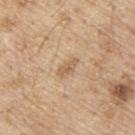workup: no biopsy performed (imaged during a skin exam) | automated metrics: an average lesion color of about L≈60 a*≈16 b*≈34 (CIELAB), a lesion–skin lightness drop of about 9, and a lesion-to-skin contrast of about 6 (normalized; higher = more distinct); a classifier nevus-likeness of about 0/100 | patient: male, aged approximately 70 | image source: ~15 mm tile from a whole-body skin photo | illumination: white-light | diameter: about 2.5 mm | body site: the upper back.